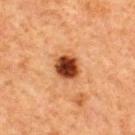{
  "biopsy_status": "not biopsied; imaged during a skin examination",
  "site": "back",
  "image": {
    "source": "total-body photography crop",
    "field_of_view_mm": 15
  },
  "lesion_size": {
    "long_diameter_mm_approx": 3.5
  },
  "patient": {
    "sex": "male",
    "age_approx": 65
  },
  "lighting": "cross-polarized",
  "automated_metrics": {
    "area_mm2_approx": 8.0,
    "eccentricity": 0.45,
    "shape_asymmetry": 0.2,
    "border_irregularity_0_10": 1.5,
    "color_variation_0_10": 6.5,
    "nevus_likeness_0_100": 100,
    "lesion_detection_confidence_0_100": 100
  }
}